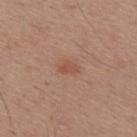{
  "biopsy_status": "not biopsied; imaged during a skin examination",
  "automated_metrics": {
    "area_mm2_approx": 3.5,
    "eccentricity": 0.8,
    "shape_asymmetry": 0.2
  },
  "patient": {
    "sex": "male",
    "age_approx": 30
  },
  "image": {
    "source": "total-body photography crop",
    "field_of_view_mm": 15
  },
  "site": "mid back"
}